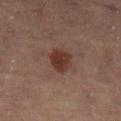Clinical impression:
No biopsy was performed on this lesion — it was imaged during a full skin examination and was not determined to be concerning.
Clinical summary:
This is a cross-polarized tile. The lesion is on the left lower leg. A 15 mm close-up tile from a total-body photography series done for melanoma screening. About 3.5 mm across. The patient is a female aged approximately 70.